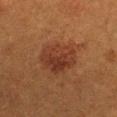Clinical impression:
Recorded during total-body skin imaging; not selected for excision or biopsy.
Image and clinical context:
A 15 mm close-up extracted from a 3D total-body photography capture. A female subject, about 40 years old. The lesion is on the chest. Longest diameter approximately 4.5 mm. This is a cross-polarized tile. The total-body-photography lesion software estimated a classifier nevus-likeness of about 70/100 and a lesion-detection confidence of about 100/100.